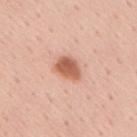  patient:
    sex: male
    age_approx: 55
  image:
    source: total-body photography crop
    field_of_view_mm: 15
  lesion_size:
    long_diameter_mm_approx: 3.5
  site: mid back